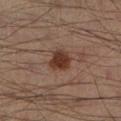Q: Was a biopsy performed?
A: total-body-photography surveillance lesion; no biopsy
Q: What is the anatomic site?
A: the left lower leg
Q: What are the patient's age and sex?
A: male, roughly 40 years of age
Q: What is the imaging modality?
A: ~15 mm tile from a whole-body skin photo